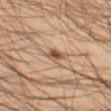workup: catalogued during a skin exam; not biopsied | image: 15 mm crop, total-body photography | patient: male, aged approximately 45 | size: ~2.5 mm (longest diameter) | site: the leg | lighting: cross-polarized illumination.